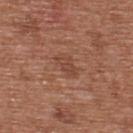Background:
The lesion is located on the upper back. A 15 mm crop from a total-body photograph taken for skin-cancer surveillance. About 2.5 mm across. Automated image analysis of the tile measured a mean CIELAB color near L≈44 a*≈23 b*≈29 and a normalized lesion–skin contrast near 5.5. The software also gave a border-irregularity index near 2.5/10 and a color-variation rating of about 0.5/10. It also reported an automated nevus-likeness rating near 0 out of 100 and lesion-presence confidence of about 100/100. The patient is a male aged 63–67. Captured under white-light illumination.